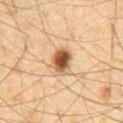{"biopsy_status": "not biopsied; imaged during a skin examination", "lighting": "cross-polarized", "site": "abdomen", "patient": {"sex": "male", "age_approx": 65}, "lesion_size": {"long_diameter_mm_approx": 3.5}, "image": {"source": "total-body photography crop", "field_of_view_mm": 15}, "automated_metrics": {"border_irregularity_0_10": 2.0, "color_variation_0_10": 6.5, "peripheral_color_asymmetry": 1.5, "nevus_likeness_0_100": 100, "lesion_detection_confidence_0_100": 100}}